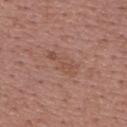biopsy status: no biopsy performed (imaged during a skin exam) | automated lesion analysis: an outline eccentricity of about 0.9 (0 = round, 1 = elongated); a classifier nevus-likeness of about 0/100 and a detector confidence of about 100 out of 100 that the crop contains a lesion | site: the upper back | imaging modality: 15 mm crop, total-body photography | size: about 4 mm | subject: female, aged approximately 50.